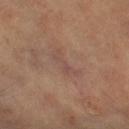Background: Cropped from a whole-body photographic skin survey; the tile spans about 15 mm. The subject is a female roughly 70 years of age. This is a cross-polarized tile. The lesion's longest dimension is about 4.5 mm. From the right thigh.